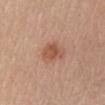workup: imaged on a skin check; not biopsied | lighting: white-light illumination | image source: total-body-photography crop, ~15 mm field of view | lesion diameter: ~3 mm (longest diameter) | anatomic site: the chest | patient: female, in their mid- to late 50s | TBP lesion metrics: a footprint of about 6.5 mm², an eccentricity of roughly 0.4, and a shape-asymmetry score of about 0.25 (0 = symmetric).